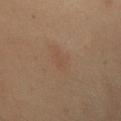follow-up — imaged on a skin check; not biopsied | subject — female, aged approximately 40 | acquisition — ~15 mm tile from a whole-body skin photo | size — ~4 mm (longest diameter) | body site — the front of the torso.